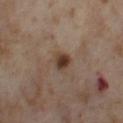On the leg.
Cropped from a whole-body photographic skin survey; the tile spans about 15 mm.
A female patient, in their mid- to late 50s.
About 3 mm across.
The total-body-photography lesion software estimated a nevus-likeness score of about 95/100.